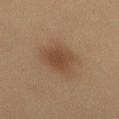Notes:
- notes · catalogued during a skin exam; not biopsied
- imaging modality · ~15 mm crop, total-body skin-cancer survey
- tile lighting · cross-polarized
- subject · male, roughly 60 years of age
- site · the mid back